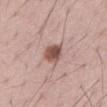biopsy status — total-body-photography surveillance lesion; no biopsy | image source — ~15 mm crop, total-body skin-cancer survey | location — the mid back | subject — male, in their 70s.